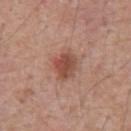Clinical impression: This lesion was catalogued during total-body skin photography and was not selected for biopsy.